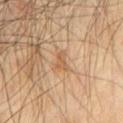The lesion was photographed on a routine skin check and not biopsied; there is no pathology result. Imaged with cross-polarized lighting. The lesion's longest dimension is about 2.5 mm. The lesion-visualizer software estimated a lesion area of about 3 mm², a shape eccentricity near 0.85, and a shape-asymmetry score of about 0.4 (0 = symmetric). The analysis additionally found a border-irregularity rating of about 4/10 and a color-variation rating of about 0/10. A region of skin cropped from a whole-body photographic capture, roughly 15 mm wide. A male patient aged 68 to 72. Located on the chest.